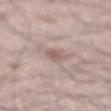workup = catalogued during a skin exam; not biopsied
lighting = white-light
location = the abdomen
automated metrics = a border-irregularity index near 2/10 and a color-variation rating of about 1.5/10
image source = ~15 mm crop, total-body skin-cancer survey
patient = male, aged around 65
lesion diameter = ~2.5 mm (longest diameter)